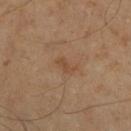This lesion was catalogued during total-body skin photography and was not selected for biopsy.
A 15 mm crop from a total-body photograph taken for skin-cancer surveillance.
Located on the right lower leg.
A male subject, approximately 55 years of age.